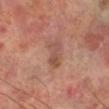Clinical impression: Part of a total-body skin-imaging series; this lesion was reviewed on a skin check and was not flagged for biopsy. Acquisition and patient details: The patient is a male aged 68 to 72. On the right lower leg. About 3.5 mm across. A 15 mm close-up tile from a total-body photography series done for melanoma screening. The tile uses cross-polarized illumination.